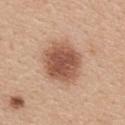| feature | finding |
|---|---|
| automated metrics | an outline eccentricity of about 0.35 (0 = round, 1 = elongated) and a symmetry-axis asymmetry near 0.15; a lesion color around L≈55 a*≈22 b*≈30 in CIELAB, a lesion–skin lightness drop of about 14, and a lesion-to-skin contrast of about 9.5 (normalized; higher = more distinct) |
| diameter | about 5.5 mm |
| body site | the upper back |
| image source | ~15 mm tile from a whole-body skin photo |
| subject | female, in their mid-40s |